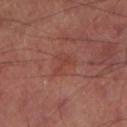workup: total-body-photography surveillance lesion; no biopsy
image-analysis metrics: a lesion area of about 4.5 mm², an outline eccentricity of about 0.75 (0 = round, 1 = elongated), and two-axis asymmetry of about 0.6; a border-irregularity index near 6/10 and internal color variation of about 1.5 on a 0–10 scale; lesion-presence confidence of about 100/100
lighting: cross-polarized
body site: the left lower leg
patient: male, approximately 65 years of age
image: ~15 mm crop, total-body skin-cancer survey